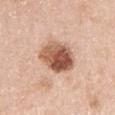• biopsy status · no biopsy performed (imaged during a skin exam)
• site · the left upper arm
• illumination · white-light illumination
• TBP lesion metrics · a border-irregularity rating of about 1.5/10 and a color-variation rating of about 9.5/10; an automated nevus-likeness rating near 85 out of 100 and lesion-presence confidence of about 100/100
• size · about 5 mm
• image source · 15 mm crop, total-body photography
• patient · female, approximately 50 years of age